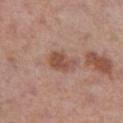biopsy_status: not biopsied; imaged during a skin examination
automated_metrics:
  vs_skin_darker_L: 10.0
  vs_skin_contrast_norm: 7.5
  border_irregularity_0_10: 2.5
  color_variation_0_10: 3.5
  peripheral_color_asymmetry: 1.0
  nevus_likeness_0_100: 10
  lesion_detection_confidence_0_100: 100
image:
  source: total-body photography crop
  field_of_view_mm: 15
lighting: white-light
site: left thigh
patient:
  sex: male
  age_approx: 70
lesion_size:
  long_diameter_mm_approx: 3.5